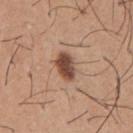workup: catalogued during a skin exam; not biopsied
subject: male, in their mid-60s
tile lighting: white-light
acquisition: total-body-photography crop, ~15 mm field of view
lesion size: about 3.5 mm
site: the front of the torso